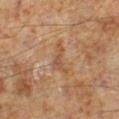biopsy_status: not biopsied; imaged during a skin examination
patient:
  sex: male
  age_approx: 60
lesion_size:
  long_diameter_mm_approx: 4.0
image:
  source: total-body photography crop
  field_of_view_mm: 15
lighting: cross-polarized
site: left lower leg